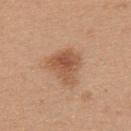The lesion's longest dimension is about 4.5 mm.
Imaged with white-light lighting.
Located on the upper back.
A roughly 15 mm field-of-view crop from a total-body skin photograph.
A female patient in their mid- to late 20s.